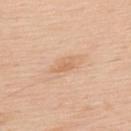Imaged during a routine full-body skin examination; the lesion was not biopsied and no histopathology is available.
The recorded lesion diameter is about 3 mm.
A male patient approximately 60 years of age.
Located on the upper back.
A roughly 15 mm field-of-view crop from a total-body skin photograph.